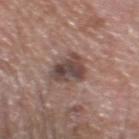imaging modality: 15 mm crop, total-body photography | illumination: white-light | subject: male, aged approximately 60 | location: the head or neck | lesion size: about 4 mm | image-analysis metrics: a lesion–skin lightness drop of about 12 and a normalized border contrast of about 9.5; an automated nevus-likeness rating near 0 out of 100 and a lesion-detection confidence of about 100/100.